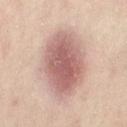Imaged during a routine full-body skin examination; the lesion was not biopsied and no histopathology is available. Cropped from a total-body skin-imaging series; the visible field is about 15 mm. The patient is a female roughly 40 years of age. The recorded lesion diameter is about 8 mm. On the abdomen. Automated image analysis of the tile measured a lesion color around L≈62 a*≈21 b*≈23 in CIELAB and a normalized lesion–skin contrast near 9. The analysis additionally found a classifier nevus-likeness of about 90/100.